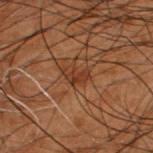- subject: male, about 50 years old
- location: the back
- illumination: cross-polarized illumination
- lesion diameter: ~3 mm (longest diameter)
- automated metrics: a lesion area of about 3.5 mm², an eccentricity of roughly 0.85, and two-axis asymmetry of about 0.45; a lesion color around L≈27 a*≈19 b*≈26 in CIELAB, about 6 CIELAB-L* units darker than the surrounding skin, and a normalized lesion–skin contrast near 7
- acquisition: total-body-photography crop, ~15 mm field of view